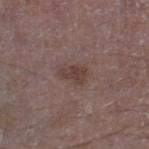workup — imaged on a skin check; not biopsied | location — the right lower leg | lesion diameter — ≈3 mm | subject — male, about 50 years old | tile lighting — white-light | image — ~15 mm tile from a whole-body skin photo.